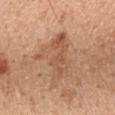Impression: Captured during whole-body skin photography for melanoma surveillance; the lesion was not biopsied. Clinical summary: On the mid back. Captured under white-light illumination. About 6 mm across. A close-up tile cropped from a whole-body skin photograph, about 15 mm across. A male patient in their mid- to late 40s.